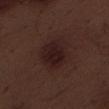Q: Was a biopsy performed?
A: no biopsy performed (imaged during a skin exam)